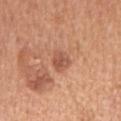Assessment: Recorded during total-body skin imaging; not selected for excision or biopsy. Clinical summary: A lesion tile, about 15 mm wide, cut from a 3D total-body photograph. Automated tile analysis of the lesion measured an area of roughly 5 mm², an outline eccentricity of about 0.7 (0 = round, 1 = elongated), and a symmetry-axis asymmetry near 0.25. The subject is a male in their mid-60s. Approximately 3 mm at its widest. Located on the mid back. Captured under white-light illumination.